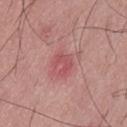Recorded during total-body skin imaging; not selected for excision or biopsy. Measured at roughly 2.5 mm in maximum diameter. A 15 mm close-up tile from a total-body photography series done for melanoma screening. A male patient, approximately 55 years of age. Located on the front of the torso.